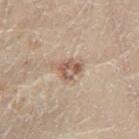notes = catalogued during a skin exam; not biopsied
acquisition = total-body-photography crop, ~15 mm field of view
illumination = cross-polarized illumination
patient = male, aged 68–72
anatomic site = the left lower leg
image-analysis metrics = an area of roughly 5.5 mm² and a symmetry-axis asymmetry near 0.3; a nevus-likeness score of about 40/100 and lesion-presence confidence of about 100/100